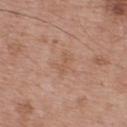  biopsy_status: not biopsied; imaged during a skin examination
  lesion_size:
    long_diameter_mm_approx: 2.5
  lighting: white-light
  patient:
    sex: male
    age_approx: 65
  image:
    source: total-body photography crop
    field_of_view_mm: 15
  site: upper back